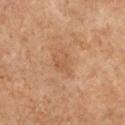Impression: Captured during whole-body skin photography for melanoma surveillance; the lesion was not biopsied. Context: Captured under cross-polarized illumination. The lesion is on the chest. Automated image analysis of the tile measured a shape eccentricity near 0.75 and two-axis asymmetry of about 0.3. And it measured a border-irregularity rating of about 3/10, a within-lesion color-variation index near 2/10, and a peripheral color-asymmetry measure near 0.5. A female subject approximately 60 years of age. Approximately 3.5 mm at its widest. A 15 mm close-up extracted from a 3D total-body photography capture.